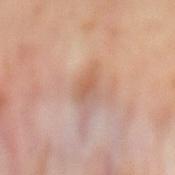<lesion>
  <biopsy_status>not biopsied; imaged during a skin examination</biopsy_status>
  <patient>
    <sex>male</sex>
    <age_approx>50</age_approx>
  </patient>
  <site>mid back</site>
  <image>
    <source>total-body photography crop</source>
    <field_of_view_mm>15</field_of_view_mm>
  </image>
</lesion>